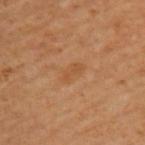| feature | finding |
|---|---|
| biopsy status | imaged on a skin check; not biopsied |
| subject | male, about 65 years old |
| image-analysis metrics | about 5 CIELAB-L* units darker than the surrounding skin and a lesion-to-skin contrast of about 4.5 (normalized; higher = more distinct); an automated nevus-likeness rating near 0 out of 100 and lesion-presence confidence of about 100/100 |
| body site | the upper back |
| imaging modality | total-body-photography crop, ~15 mm field of view |
| tile lighting | cross-polarized |